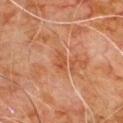<case>
  <biopsy_status>not biopsied; imaged during a skin examination</biopsy_status>
  <patient>
    <sex>male</sex>
    <age_approx>80</age_approx>
  </patient>
  <image>
    <source>total-body photography crop</source>
    <field_of_view_mm>15</field_of_view_mm>
  </image>
  <lesion_size>
    <long_diameter_mm_approx>2.5</long_diameter_mm_approx>
  </lesion_size>
  <lighting>cross-polarized</lighting>
  <site>front of the torso</site>
  <automated_metrics>
    <area_mm2_approx>3.0</area_mm2_approx>
    <shape_asymmetry>0.3</shape_asymmetry>
    <cielab_L>40</cielab_L>
    <cielab_a>23</cielab_a>
    <cielab_b>31</cielab_b>
    <vs_skin_contrast_norm>6.0</vs_skin_contrast_norm>
    <nevus_likeness_0_100>0</nevus_likeness_0_100>
    <lesion_detection_confidence_0_100>100</lesion_detection_confidence_0_100>
  </automated_metrics>
</case>